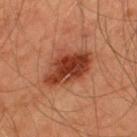The lesion was photographed on a routine skin check and not biopsied; there is no pathology result. A male patient aged approximately 45. A roughly 15 mm field-of-view crop from a total-body skin photograph. Located on the upper back. Captured under cross-polarized illumination. About 6 mm across.